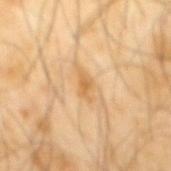Clinical impression:
The lesion was tiled from a total-body skin photograph and was not biopsied.
Clinical summary:
The patient is a male aged approximately 65. The lesion is located on the mid back. A close-up tile cropped from a whole-body skin photograph, about 15 mm across.